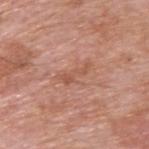{"site": "back", "patient": {"sex": "male", "age_approx": 60}, "image": {"source": "total-body photography crop", "field_of_view_mm": 15}}